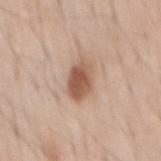Imaged during a routine full-body skin examination; the lesion was not biopsied and no histopathology is available. Imaged with white-light lighting. From the mid back. A lesion tile, about 15 mm wide, cut from a 3D total-body photograph. A male subject, aged 58 to 62. An algorithmic analysis of the crop reported a lesion area of about 8.5 mm². The analysis additionally found a lesion color around L≈56 a*≈19 b*≈30 in CIELAB, about 14 CIELAB-L* units darker than the surrounding skin, and a normalized border contrast of about 9. And it measured a border-irregularity rating of about 2/10.